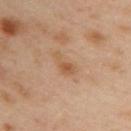Q: Was a biopsy performed?
A: total-body-photography surveillance lesion; no biopsy
Q: What kind of image is this?
A: ~15 mm tile from a whole-body skin photo
Q: What did automated image analysis measure?
A: a lesion area of about 4 mm², an eccentricity of roughly 0.9, and two-axis asymmetry of about 0.45; a lesion color around L≈57 a*≈20 b*≈35 in CIELAB, roughly 8 lightness units darker than nearby skin, and a lesion-to-skin contrast of about 6 (normalized; higher = more distinct); a border-irregularity rating of about 4.5/10 and a color-variation rating of about 3/10
Q: What lighting was used for the tile?
A: cross-polarized
Q: What are the patient's age and sex?
A: female, in their 40s
Q: Where on the body is the lesion?
A: the upper back